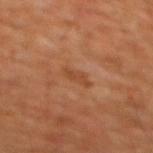No biopsy was performed on this lesion — it was imaged during a full skin examination and was not determined to be concerning. Captured under cross-polarized illumination. Automated tile analysis of the lesion measured a border-irregularity index near 4/10, internal color variation of about 1 on a 0–10 scale, and peripheral color asymmetry of about 0.5. Cropped from a total-body skin-imaging series; the visible field is about 15 mm. Located on the chest. A male patient aged around 60. The recorded lesion diameter is about 3 mm.